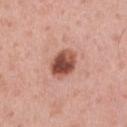• notes: catalogued during a skin exam; not biopsied
• imaging modality: 15 mm crop, total-body photography
• TBP lesion metrics: a footprint of about 8.5 mm² and an eccentricity of roughly 0.6; a mean CIELAB color near L≈51 a*≈25 b*≈28 and a lesion-to-skin contrast of about 11 (normalized; higher = more distinct); an automated nevus-likeness rating near 75 out of 100 and lesion-presence confidence of about 100/100
• subject: male, aged 58–62
• lesion size: about 3.5 mm
• anatomic site: the front of the torso